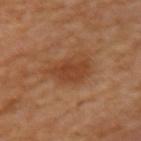Captured during whole-body skin photography for melanoma surveillance; the lesion was not biopsied. A 15 mm crop from a total-body photograph taken for skin-cancer surveillance. On the left upper arm. The recorded lesion diameter is about 5 mm. The patient is a female in their mid- to late 50s.